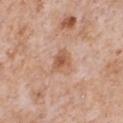Part of a total-body skin-imaging series; this lesion was reviewed on a skin check and was not flagged for biopsy.
A roughly 15 mm field-of-view crop from a total-body skin photograph.
The subject is a male approximately 65 years of age.
The lesion is on the chest.
Measured at roughly 3 mm in maximum diameter.
The lesion-visualizer software estimated a lesion area of about 5 mm² and an outline eccentricity of about 0.6 (0 = round, 1 = elongated). And it measured a border-irregularity index near 2.5/10, a color-variation rating of about 2.5/10, and a peripheral color-asymmetry measure near 0.5. And it measured a nevus-likeness score of about 0/100.